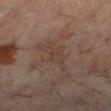Impression:
This lesion was catalogued during total-body skin photography and was not selected for biopsy.
Image and clinical context:
The patient is a female aged around 60. A close-up tile cropped from a whole-body skin photograph, about 15 mm across. Longest diameter approximately 5.5 mm. The lesion is located on the left lower leg. The tile uses cross-polarized illumination.